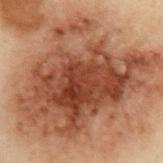Findings:
– workup: catalogued during a skin exam; not biopsied
– subject: male, about 55 years old
– site: the left upper arm
– image source: ~15 mm tile from a whole-body skin photo
– tile lighting: cross-polarized illumination
– automated metrics: an area of roughly 95 mm², a shape eccentricity near 0.75, and a symmetry-axis asymmetry near 0.4; a lesion color around L≈39 a*≈20 b*≈27 in CIELAB, a lesion–skin lightness drop of about 15, and a lesion-to-skin contrast of about 12 (normalized; higher = more distinct)